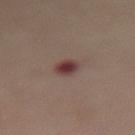Findings:
• biopsy status · catalogued during a skin exam; not biopsied
• tile lighting · cross-polarized illumination
• acquisition · ~15 mm tile from a whole-body skin photo
• anatomic site · the front of the torso
• lesion size · ≈2.5 mm
• subject · female, aged around 50